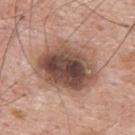The lesion was photographed on a routine skin check and not biopsied; there is no pathology result.
The lesion is located on the upper back.
Cropped from a whole-body photographic skin survey; the tile spans about 15 mm.
A male subject, aged approximately 60.
Imaged with white-light lighting.
Longest diameter approximately 7 mm.